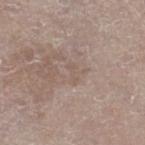Clinical impression: This lesion was catalogued during total-body skin photography and was not selected for biopsy. Background: The recorded lesion diameter is about 2.5 mm. The lesion is located on the right lower leg. A male patient aged approximately 80. Cropped from a whole-body photographic skin survey; the tile spans about 15 mm. Captured under white-light illumination. The total-body-photography lesion software estimated an average lesion color of about L≈55 a*≈14 b*≈23 (CIELAB) and a normalized border contrast of about 3.5. The software also gave a border-irregularity rating of about 4.5/10, a color-variation rating of about 0/10, and peripheral color asymmetry of about 0. It also reported an automated nevus-likeness rating near 0 out of 100 and lesion-presence confidence of about 70/100.